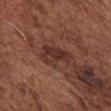| feature | finding |
|---|---|
| notes | catalogued during a skin exam; not biopsied |
| imaging modality | ~15 mm crop, total-body skin-cancer survey |
| anatomic site | the chest |
| patient | male, aged around 75 |
| lighting | white-light illumination |
| automated metrics | a lesion color around L≈33 a*≈21 b*≈24 in CIELAB, about 9 CIELAB-L* units darker than the surrounding skin, and a normalized lesion–skin contrast near 8.5; a classifier nevus-likeness of about 5/100 and a detector confidence of about 95 out of 100 that the crop contains a lesion |
| lesion size | about 4.5 mm |